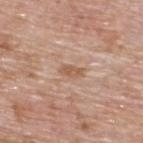Q: Is there a histopathology result?
A: catalogued during a skin exam; not biopsied